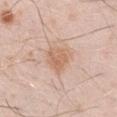No biopsy was performed on this lesion — it was imaged during a full skin examination and was not determined to be concerning.
An algorithmic analysis of the crop reported a footprint of about 7.5 mm², an eccentricity of roughly 0.4, and a shape-asymmetry score of about 0.25 (0 = symmetric). The software also gave a border-irregularity index near 2.5/10, internal color variation of about 3.5 on a 0–10 scale, and radial color variation of about 1. The analysis additionally found an automated nevus-likeness rating near 45 out of 100 and a detector confidence of about 100 out of 100 that the crop contains a lesion.
Imaged with white-light lighting.
A 15 mm crop from a total-body photograph taken for skin-cancer surveillance.
A male patient about 30 years old.
The lesion is located on the arm.